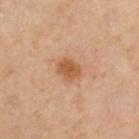workup: imaged on a skin check; not biopsied
lesion diameter: ≈2.5 mm
image-analysis metrics: a border-irregularity index near 2/10, internal color variation of about 2.5 on a 0–10 scale, and peripheral color asymmetry of about 1; a lesion-detection confidence of about 100/100
acquisition: ~15 mm tile from a whole-body skin photo
anatomic site: the left thigh
illumination: cross-polarized
subject: female, about 55 years old